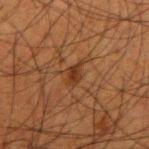Recorded during total-body skin imaging; not selected for excision or biopsy. Measured at roughly 3 mm in maximum diameter. This is a cross-polarized tile. A roughly 15 mm field-of-view crop from a total-body skin photograph. The lesion is on the right upper arm. A male subject, in their 50s.